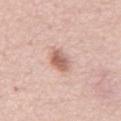Recorded during total-body skin imaging; not selected for excision or biopsy. The recorded lesion diameter is about 3 mm. A 15 mm close-up tile from a total-body photography series done for melanoma screening. The patient is a male in their mid- to late 50s.